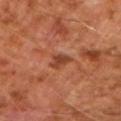biopsy status: imaged on a skin check; not biopsied
size: about 2.5 mm
subject: male, in their 60s
site: the right forearm
image source: ~15 mm tile from a whole-body skin photo
tile lighting: cross-polarized